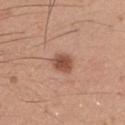Assessment:
Imaged during a routine full-body skin examination; the lesion was not biopsied and no histopathology is available.
Background:
Imaged with white-light lighting. A male subject, aged around 20. Cropped from a whole-body photographic skin survey; the tile spans about 15 mm. Located on the right upper arm. About 3 mm across.